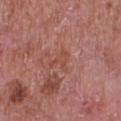This lesion was catalogued during total-body skin photography and was not selected for biopsy.
Cropped from a whole-body photographic skin survey; the tile spans about 15 mm.
A male patient roughly 65 years of age.
Imaged with white-light lighting.
The lesion is on the chest.